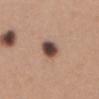Part of a total-body skin-imaging series; this lesion was reviewed on a skin check and was not flagged for biopsy.